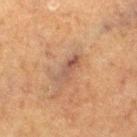<lesion>
<biopsy_status>not biopsied; imaged during a skin examination</biopsy_status>
<image>
  <source>total-body photography crop</source>
  <field_of_view_mm>15</field_of_view_mm>
</image>
<site>leg</site>
<lighting>cross-polarized</lighting>
<lesion_size>
  <long_diameter_mm_approx>4.0</long_diameter_mm_approx>
</lesion_size>
<patient>
  <sex>male</sex>
  <age_approx>75</age_approx>
</patient>
<automated_metrics>
  <shape_asymmetry>0.35</shape_asymmetry>
</automated_metrics>
</lesion>